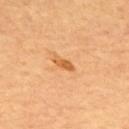Clinical impression:
Captured during whole-body skin photography for melanoma surveillance; the lesion was not biopsied.
Background:
Automated image analysis of the tile measured a lesion area of about 3 mm² and a shape-asymmetry score of about 0.2 (0 = symmetric). It also reported an average lesion color of about L≈59 a*≈25 b*≈44 (CIELAB) and about 10 CIELAB-L* units darker than the surrounding skin. The software also gave a color-variation rating of about 0.5/10 and peripheral color asymmetry of about 0. The analysis additionally found a classifier nevus-likeness of about 40/100 and a detector confidence of about 100 out of 100 that the crop contains a lesion. A male subject, roughly 60 years of age. This is a cross-polarized tile. A lesion tile, about 15 mm wide, cut from a 3D total-body photograph. The lesion is on the upper back.